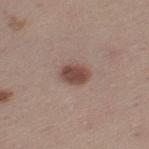This lesion was catalogued during total-body skin photography and was not selected for biopsy. On the left thigh. Cropped from a whole-body photographic skin survey; the tile spans about 15 mm. A female patient aged 43–47. Longest diameter approximately 3.5 mm. The tile uses white-light illumination.